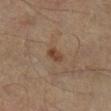Part of a total-body skin-imaging series; this lesion was reviewed on a skin check and was not flagged for biopsy. A roughly 15 mm field-of-view crop from a total-body skin photograph. The lesion-visualizer software estimated a footprint of about 3.5 mm² and two-axis asymmetry of about 0.3. The software also gave an automated nevus-likeness rating near 60 out of 100 and a detector confidence of about 100 out of 100 that the crop contains a lesion. Imaged with cross-polarized lighting. A male subject, aged around 55. On the left leg.